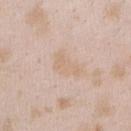This lesion was catalogued during total-body skin photography and was not selected for biopsy. The lesion is on the arm. A female subject aged approximately 25. The lesion-visualizer software estimated a lesion area of about 5.5 mm², an outline eccentricity of about 0.85 (0 = round, 1 = elongated), and two-axis asymmetry of about 0.5. And it measured border irregularity of about 5.5 on a 0–10 scale, a color-variation rating of about 1.5/10, and a peripheral color-asymmetry measure near 0.5. Measured at roughly 3.5 mm in maximum diameter. A 15 mm close-up tile from a total-body photography series done for melanoma screening.